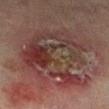workup — imaged on a skin check; not biopsied
site — the left lower leg
image-analysis metrics — a symmetry-axis asymmetry near 0.3; a peripheral color-asymmetry measure near 3.5; lesion-presence confidence of about 100/100
size — ~8.5 mm (longest diameter)
subject — male, in their mid- to late 70s
imaging modality — ~15 mm tile from a whole-body skin photo